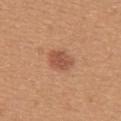The lesion was tiled from a total-body skin photograph and was not biopsied. An algorithmic analysis of the crop reported a lesion area of about 6 mm², a shape eccentricity near 0.75, and a shape-asymmetry score of about 0.2 (0 = symmetric). And it measured a lesion color around L≈53 a*≈24 b*≈33 in CIELAB and a lesion-to-skin contrast of about 7.5 (normalized; higher = more distinct). The analysis additionally found a color-variation rating of about 2/10. The analysis additionally found a classifier nevus-likeness of about 80/100 and lesion-presence confidence of about 100/100. Captured under white-light illumination. The lesion is on the upper back. Approximately 3.5 mm at its widest. A roughly 15 mm field-of-view crop from a total-body skin photograph. A female patient aged around 30.